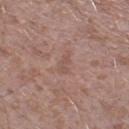Clinical impression: No biopsy was performed on this lesion — it was imaged during a full skin examination and was not determined to be concerning. Image and clinical context: The subject is a male aged 43–47. A lesion tile, about 15 mm wide, cut from a 3D total-body photograph. Located on the leg. Automated tile analysis of the lesion measured a mean CIELAB color near L≈52 a*≈19 b*≈25, about 6 CIELAB-L* units darker than the surrounding skin, and a normalized lesion–skin contrast near 4.5. The lesion's longest dimension is about 2.5 mm.